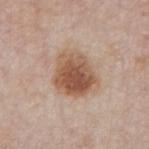No biopsy was performed on this lesion — it was imaged during a full skin examination and was not determined to be concerning. A male patient, aged 73–77. Located on the mid back. Imaged with white-light lighting. An algorithmic analysis of the crop reported a footprint of about 18 mm² and a shape-asymmetry score of about 0.2 (0 = symmetric). The analysis additionally found a lesion color around L≈55 a*≈19 b*≈30 in CIELAB, roughly 13 lightness units darker than nearby skin, and a lesion-to-skin contrast of about 9.5 (normalized; higher = more distinct). The analysis additionally found an automated nevus-likeness rating near 100 out of 100 and a lesion-detection confidence of about 100/100. A region of skin cropped from a whole-body photographic capture, roughly 15 mm wide.